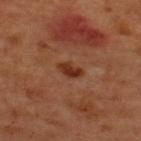The lesion was tiled from a total-body skin photograph and was not biopsied. Automated image analysis of the tile measured an area of roughly 4 mm², an outline eccentricity of about 0.8 (0 = round, 1 = elongated), and a shape-asymmetry score of about 0.3 (0 = symmetric). And it measured an average lesion color of about L≈34 a*≈25 b*≈32 (CIELAB), a lesion–skin lightness drop of about 10, and a lesion-to-skin contrast of about 9 (normalized; higher = more distinct). And it measured border irregularity of about 3 on a 0–10 scale, a color-variation rating of about 2/10, and peripheral color asymmetry of about 1. The patient is a male about 50 years old. A roughly 15 mm field-of-view crop from a total-body skin photograph. Located on the upper back. This is a cross-polarized tile.